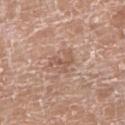* biopsy status: catalogued during a skin exam; not biopsied
* diameter: about 3 mm
* acquisition: 15 mm crop, total-body photography
* anatomic site: the right lower leg
* patient: female, in their mid-70s
* tile lighting: white-light